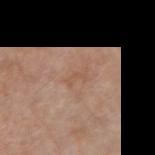<case>
<biopsy_status>not biopsied; imaged during a skin examination</biopsy_status>
<site>right upper arm</site>
<patient>
  <sex>male</sex>
  <age_approx>80</age_approx>
</patient>
<lighting>white-light</lighting>
<image>
  <source>total-body photography crop</source>
  <field_of_view_mm>15</field_of_view_mm>
</image>
<lesion_size>
  <long_diameter_mm_approx>2.5</long_diameter_mm_approx>
</lesion_size>
</case>